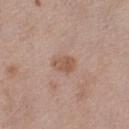Q: Where on the body is the lesion?
A: the left thigh
Q: Patient demographics?
A: female, roughly 40 years of age
Q: How was this image acquired?
A: ~15 mm tile from a whole-body skin photo